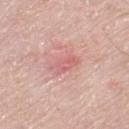No biopsy was performed on this lesion — it was imaged during a full skin examination and was not determined to be concerning.
This is a white-light tile.
The lesion is located on the left thigh.
Approximately 4 mm at its widest.
This image is a 15 mm lesion crop taken from a total-body photograph.
A male subject, approximately 80 years of age.
Automated image analysis of the tile measured a border-irregularity rating of about 3/10, a color-variation rating of about 2.5/10, and a peripheral color-asymmetry measure near 1. The analysis additionally found an automated nevus-likeness rating near 0 out of 100.